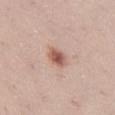Clinical impression: Part of a total-body skin-imaging series; this lesion was reviewed on a skin check and was not flagged for biopsy. Acquisition and patient details: About 3 mm across. The total-body-photography lesion software estimated an eccentricity of roughly 0.7 and a shape-asymmetry score of about 0.2 (0 = symmetric). And it measured a lesion color around L≈57 a*≈21 b*≈27 in CIELAB and a normalized lesion–skin contrast near 8.5. On the left thigh. The subject is a female aged approximately 30. A roughly 15 mm field-of-view crop from a total-body skin photograph.